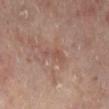| feature | finding |
|---|---|
| notes | no biopsy performed (imaged during a skin exam) |
| tile lighting | cross-polarized |
| automated metrics | a lesion area of about 3 mm² and an eccentricity of roughly 0.95; a classifier nevus-likeness of about 0/100 and lesion-presence confidence of about 100/100 |
| lesion diameter | ~3.5 mm (longest diameter) |
| patient | male, about 65 years old |
| body site | the left lower leg |
| acquisition | total-body-photography crop, ~15 mm field of view |